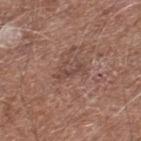Part of a total-body skin-imaging series; this lesion was reviewed on a skin check and was not flagged for biopsy. An algorithmic analysis of the crop reported a footprint of about 4.5 mm², a shape eccentricity near 0.85, and two-axis asymmetry of about 0.35. And it measured an average lesion color of about L≈46 a*≈19 b*≈24 (CIELAB), roughly 7 lightness units darker than nearby skin, and a lesion-to-skin contrast of about 5.5 (normalized; higher = more distinct). The software also gave a within-lesion color-variation index near 3.5/10 and peripheral color asymmetry of about 1.5. A male patient, in their 80s. A close-up tile cropped from a whole-body skin photograph, about 15 mm across. This is a white-light tile. The lesion is on the leg. Measured at roughly 3.5 mm in maximum diameter.